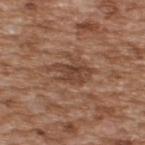This lesion was catalogued during total-body skin photography and was not selected for biopsy.
The lesion is located on the back.
Imaged with white-light lighting.
A male subject in their mid-60s.
Cropped from a total-body skin-imaging series; the visible field is about 15 mm.
The lesion's longest dimension is about 5 mm.